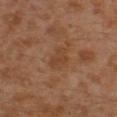| feature | finding |
|---|---|
| notes | total-body-photography surveillance lesion; no biopsy |
| site | the leg |
| size | ≈3 mm |
| lighting | cross-polarized |
| image | 15 mm crop, total-body photography |
| subject | male, roughly 30 years of age |